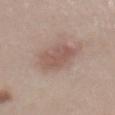No biopsy was performed on this lesion — it was imaged during a full skin examination and was not determined to be concerning.